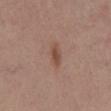Clinical impression:
Part of a total-body skin-imaging series; this lesion was reviewed on a skin check and was not flagged for biopsy.
Background:
A female subject roughly 50 years of age. The lesion is on the chest. A region of skin cropped from a whole-body photographic capture, roughly 15 mm wide.